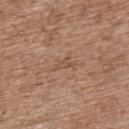Notes:
– notes · catalogued during a skin exam; not biopsied